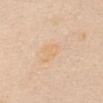<lesion>
<automated_metrics>
  <area_mm2_approx>4.0</area_mm2_approx>
  <eccentricity>0.75</eccentricity>
  <shape_asymmetry>0.4</shape_asymmetry>
  <border_irregularity_0_10>4.0</border_irregularity_0_10>
  <color_variation_0_10>1.5</color_variation_0_10>
  <peripheral_color_asymmetry>0.5</peripheral_color_asymmetry>
  <nevus_likeness_0_100>0</nevus_likeness_0_100>
  <lesion_detection_confidence_0_100>100</lesion_detection_confidence_0_100>
</automated_metrics>
<lighting>white-light</lighting>
<patient>
  <sex>female</sex>
  <age_approx>55</age_approx>
</patient>
<site>abdomen</site>
<image>
  <source>total-body photography crop</source>
  <field_of_view_mm>15</field_of_view_mm>
</image>
<lesion_size>
  <long_diameter_mm_approx>2.5</long_diameter_mm_approx>
</lesion_size>
</lesion>